From the left upper arm; a 15 mm close-up tile from a total-body photography series done for melanoma screening; a male patient, aged approximately 40; Automated tile analysis of the lesion measured an area of roughly 3.5 mm², an outline eccentricity of about 0.6 (0 = round, 1 = elongated), and two-axis asymmetry of about 0.4. And it measured a normalized border contrast of about 11.5. The analysis additionally found a classifier nevus-likeness of about 95/100 and lesion-presence confidence of about 100/100: 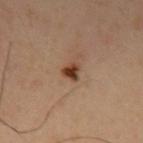Conclusion:
The biopsy diagnosis was a seborrheic keratosis (benign).